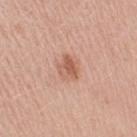acquisition: total-body-photography crop, ~15 mm field of view
subject: female, roughly 50 years of age
site: the right upper arm